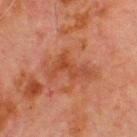follow-up = imaged on a skin check; not biopsied | image source = ~15 mm crop, total-body skin-cancer survey | anatomic site = the chest | illumination = cross-polarized | patient = male, about 70 years old.